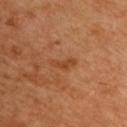Imaged during a routine full-body skin examination; the lesion was not biopsied and no histopathology is available. A male patient, about 50 years old. This image is a 15 mm lesion crop taken from a total-body photograph. The lesion's longest dimension is about 3.5 mm. The lesion is on the chest. The lesion-visualizer software estimated a footprint of about 4 mm², an eccentricity of roughly 0.9, and a shape-asymmetry score of about 0.5 (0 = symmetric). And it measured a within-lesion color-variation index near 1/10. Captured under cross-polarized illumination.